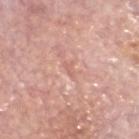Q: Was this lesion biopsied?
A: no biopsy performed (imaged during a skin exam)
Q: How was this image acquired?
A: ~15 mm tile from a whole-body skin photo
Q: How was the tile lit?
A: white-light illumination
Q: What are the patient's age and sex?
A: male, aged 58 to 62
Q: What is the anatomic site?
A: the head or neck
Q: Lesion size?
A: ~2 mm (longest diameter)
Q: What did automated image analysis measure?
A: a lesion area of about 1 mm², a shape eccentricity near 0.9, and two-axis asymmetry of about 0.35; a mean CIELAB color near L≈63 a*≈24 b*≈27 and about 7 CIELAB-L* units darker than the surrounding skin; border irregularity of about 4 on a 0–10 scale and a color-variation rating of about 0/10; a classifier nevus-likeness of about 0/100 and lesion-presence confidence of about 30/100